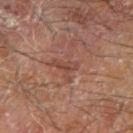Q: Was a biopsy performed?
A: catalogued during a skin exam; not biopsied